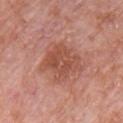<record>
<biopsy_status>not biopsied; imaged during a skin examination</biopsy_status>
<lesion_size>
  <long_diameter_mm_approx>5.0</long_diameter_mm_approx>
</lesion_size>
<patient>
  <sex>male</sex>
  <age_approx>80</age_approx>
</patient>
<lighting>white-light</lighting>
<image>
  <source>total-body photography crop</source>
  <field_of_view_mm>15</field_of_view_mm>
</image>
<site>chest</site>
</record>